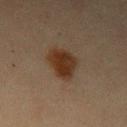Part of a total-body skin-imaging series; this lesion was reviewed on a skin check and was not flagged for biopsy.